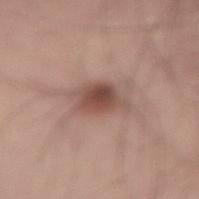Findings:
* follow-up · total-body-photography surveillance lesion; no biopsy
* anatomic site · the back
* illumination · white-light
* TBP lesion metrics · an area of roughly 9 mm², a shape eccentricity near 0.8, and two-axis asymmetry of about 0.35; a border-irregularity index near 3.5/10, a color-variation rating of about 5/10, and peripheral color asymmetry of about 1.5; an automated nevus-likeness rating near 90 out of 100
* imaging modality · ~15 mm crop, total-body skin-cancer survey
* lesion diameter · ≈4.5 mm
* subject · male, in their 70s